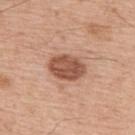{"lesion_size": {"long_diameter_mm_approx": 4.5}, "automated_metrics": {"nevus_likeness_0_100": 35, "lesion_detection_confidence_0_100": 100}, "image": {"source": "total-body photography crop", "field_of_view_mm": 15}, "site": "upper back", "patient": {"sex": "male", "age_approx": 55}}